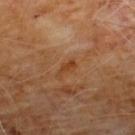• biopsy status · imaged on a skin check; not biopsied
• patient · male, aged around 60
• site · the chest
• image · ~15 mm crop, total-body skin-cancer survey